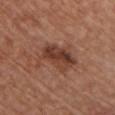No biopsy was performed on this lesion — it was imaged during a full skin examination and was not determined to be concerning. A male subject aged around 65. The lesion's longest dimension is about 5.5 mm. Automated image analysis of the tile measured an area of roughly 14 mm², an eccentricity of roughly 0.7, and a symmetry-axis asymmetry near 0.35. The lesion is on the front of the torso. A lesion tile, about 15 mm wide, cut from a 3D total-body photograph.